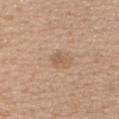Impression: No biopsy was performed on this lesion — it was imaged during a full skin examination and was not determined to be concerning. Acquisition and patient details: The tile uses white-light illumination. Longest diameter approximately 2.5 mm. On the upper back. A female patient, in their mid- to late 20s. A lesion tile, about 15 mm wide, cut from a 3D total-body photograph.